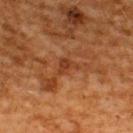- notes: imaged on a skin check; not biopsied
- image-analysis metrics: a footprint of about 3 mm² and an outline eccentricity of about 0.65 (0 = round, 1 = elongated); about 7 CIELAB-L* units darker than the surrounding skin and a normalized lesion–skin contrast near 7; a border-irregularity rating of about 4/10 and a peripheral color-asymmetry measure near 1
- tile lighting: cross-polarized
- size: ≈2.5 mm
- acquisition: 15 mm crop, total-body photography
- patient: male, approximately 60 years of age
- anatomic site: the upper back